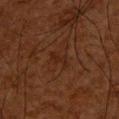Q: What is the anatomic site?
A: the upper back
Q: How was this image acquired?
A: 15 mm crop, total-body photography
Q: What are the patient's age and sex?
A: male, in their mid-60s